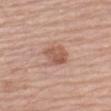Context:
A 15 mm close-up extracted from a 3D total-body photography capture. Captured under white-light illumination. Located on the left upper arm. The recorded lesion diameter is about 3.5 mm. A male patient, in their mid-70s.